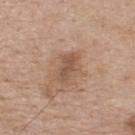{
  "biopsy_status": "not biopsied; imaged during a skin examination",
  "image": {
    "source": "total-body photography crop",
    "field_of_view_mm": 15
  },
  "site": "upper back",
  "patient": {
    "sex": "male",
    "age_approx": 75
  }
}